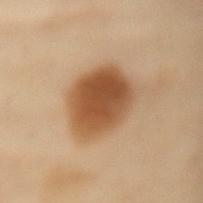Impression: Captured during whole-body skin photography for melanoma surveillance; the lesion was not biopsied. Image and clinical context: A female subject, aged approximately 60. About 6.5 mm across. This image is a 15 mm lesion crop taken from a total-body photograph. Automated tile analysis of the lesion measured an area of roughly 23 mm², an eccentricity of roughly 0.7, and a symmetry-axis asymmetry near 0.15. The software also gave a mean CIELAB color near L≈45 a*≈18 b*≈32, roughly 13 lightness units darker than nearby skin, and a normalized lesion–skin contrast near 10.5. It also reported a classifier nevus-likeness of about 100/100 and lesion-presence confidence of about 100/100. Located on the mid back.